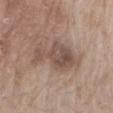Clinical impression:
The lesion was photographed on a routine skin check and not biopsied; there is no pathology result.